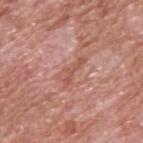Captured during whole-body skin photography for melanoma surveillance; the lesion was not biopsied.
About 4 mm across.
A male subject, roughly 60 years of age.
A 15 mm close-up tile from a total-body photography series done for melanoma screening.
On the back.